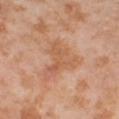Impression: No biopsy was performed on this lesion — it was imaged during a full skin examination and was not determined to be concerning. Clinical summary: Longest diameter approximately 6 mm. A lesion tile, about 15 mm wide, cut from a 3D total-body photograph. A female patient, in their mid- to late 50s. The lesion is located on the right thigh.